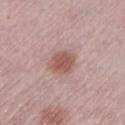Assessment: No biopsy was performed on this lesion — it was imaged during a full skin examination and was not determined to be concerning. Acquisition and patient details: The lesion is located on the left lower leg. A lesion tile, about 15 mm wide, cut from a 3D total-body photograph. A female patient approximately 65 years of age. About 3.5 mm across. This is a white-light tile.